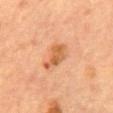Findings:
- biopsy status — total-body-photography surveillance lesion; no biopsy
- automated metrics — a mean CIELAB color near L≈54 a*≈24 b*≈37, about 10 CIELAB-L* units darker than the surrounding skin, and a lesion-to-skin contrast of about 7.5 (normalized; higher = more distinct); a nevus-likeness score of about 30/100 and lesion-presence confidence of about 100/100
- size — ~4 mm (longest diameter)
- body site — the abdomen
- acquisition — ~15 mm crop, total-body skin-cancer survey
- patient — female, aged approximately 60